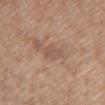The lesion was tiled from a total-body skin photograph and was not biopsied. Approximately 3 mm at its widest. A 15 mm close-up extracted from a 3D total-body photography capture. Located on the front of the torso. Imaged with white-light lighting. A female subject aged 63 to 67. The lesion-visualizer software estimated a footprint of about 4.5 mm², a shape eccentricity near 0.75, and two-axis asymmetry of about 0.35. The analysis additionally found border irregularity of about 3.5 on a 0–10 scale and a color-variation rating of about 2/10.